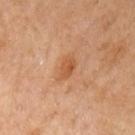notes: total-body-photography surveillance lesion; no biopsy | automated lesion analysis: an area of roughly 4.5 mm² and a shape-asymmetry score of about 0.25 (0 = symmetric); a classifier nevus-likeness of about 60/100 and a lesion-detection confidence of about 100/100 | imaging modality: total-body-photography crop, ~15 mm field of view | site: the left upper arm | lesion size: ≈3 mm | lighting: cross-polarized illumination | subject: female, approximately 60 years of age.